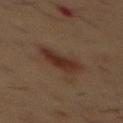Part of a total-body skin-imaging series; this lesion was reviewed on a skin check and was not flagged for biopsy. Located on the chest. A male subject roughly 55 years of age. The lesion-visualizer software estimated an area of roughly 9.5 mm² and two-axis asymmetry of about 0.35. And it measured a lesion–skin lightness drop of about 8. This is a cross-polarized tile. A region of skin cropped from a whole-body photographic capture, roughly 15 mm wide.